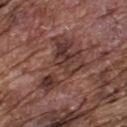This lesion was catalogued during total-body skin photography and was not selected for biopsy. A region of skin cropped from a whole-body photographic capture, roughly 15 mm wide. A male patient aged approximately 75. Located on the upper back. Imaged with white-light lighting. Automated tile analysis of the lesion measured a lesion area of about 22 mm² and a symmetry-axis asymmetry near 0.55. The software also gave a mean CIELAB color near L≈37 a*≈20 b*≈22, about 9 CIELAB-L* units darker than the surrounding skin, and a normalized border contrast of about 7.5. And it measured a within-lesion color-variation index near 7/10 and radial color variation of about 2.5. It also reported a classifier nevus-likeness of about 0/100 and a lesion-detection confidence of about 100/100.